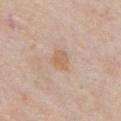Q: Where on the body is the lesion?
A: the front of the torso
Q: Automated lesion metrics?
A: an average lesion color of about L≈63 a*≈17 b*≈32 (CIELAB) and about 7 CIELAB-L* units darker than the surrounding skin; a border-irregularity rating of about 2/10 and a color-variation rating of about 1.5/10; a detector confidence of about 100 out of 100 that the crop contains a lesion
Q: How was this image acquired?
A: ~15 mm crop, total-body skin-cancer survey
Q: What are the patient's age and sex?
A: male, aged 73 to 77
Q: Illumination type?
A: white-light
Q: Lesion size?
A: ~3 mm (longest diameter)